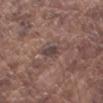Recorded during total-body skin imaging; not selected for excision or biopsy.
Imaged with white-light lighting.
Cropped from a whole-body photographic skin survey; the tile spans about 15 mm.
A male patient in their mid-70s.
Automated image analysis of the tile measured a lesion color around L≈42 a*≈15 b*≈17 in CIELAB and roughly 8 lightness units darker than nearby skin. The software also gave a classifier nevus-likeness of about 0/100.
Measured at roughly 3.5 mm in maximum diameter.
Located on the left forearm.